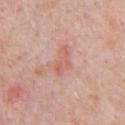The lesion was tiled from a total-body skin photograph and was not biopsied. This image is a 15 mm lesion crop taken from a total-body photograph. Longest diameter approximately 3.5 mm. From the chest. Automated image analysis of the tile measured a lesion area of about 4 mm², a shape eccentricity near 0.9, and a symmetry-axis asymmetry near 0.5. It also reported an average lesion color of about L≈61 a*≈27 b*≈27 (CIELAB) and about 8 CIELAB-L* units darker than the surrounding skin. It also reported border irregularity of about 6 on a 0–10 scale, internal color variation of about 1.5 on a 0–10 scale, and radial color variation of about 0. A male patient in their 50s. Imaged with white-light lighting.